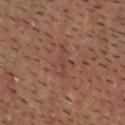biopsy_status: not biopsied; imaged during a skin examination
image:
  source: total-body photography crop
  field_of_view_mm: 15
automated_metrics:
  area_mm2_approx: 6.5
  eccentricity: 0.7
  shape_asymmetry: 0.45
  cielab_L: 41
  cielab_a: 21
  cielab_b: 23
  vs_skin_darker_L: 5.0
  vs_skin_contrast_norm: 4.5
  border_irregularity_0_10: 5.5
  lesion_detection_confidence_0_100: 95
lesion_size:
  long_diameter_mm_approx: 3.5
site: head or neck
patient:
  sex: male
  age_approx: 60
lighting: cross-polarized